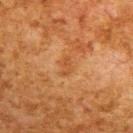The lesion was photographed on a routine skin check and not biopsied; there is no pathology result.
The lesion is located on the back.
A lesion tile, about 15 mm wide, cut from a 3D total-body photograph.
This is a cross-polarized tile.
Approximately 2.5 mm at its widest.
A male subject aged 78–82.